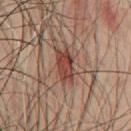workup: catalogued during a skin exam; not biopsied | subject: male, aged 58 to 62 | automated metrics: an area of roughly 7.5 mm², a shape eccentricity near 0.9, and a symmetry-axis asymmetry near 0.25; an automated nevus-likeness rating near 20 out of 100 and a lesion-detection confidence of about 100/100 | lesion size: about 4.5 mm | imaging modality: total-body-photography crop, ~15 mm field of view | site: the chest | lighting: cross-polarized illumination.